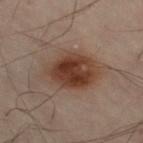notes=imaged on a skin check; not biopsied
anatomic site=the left leg
acquisition=~15 mm crop, total-body skin-cancer survey
patient=male, about 50 years old